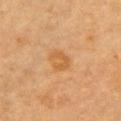Captured during whole-body skin photography for melanoma surveillance; the lesion was not biopsied. A roughly 15 mm field-of-view crop from a total-body skin photograph. The lesion is on the chest. This is a cross-polarized tile. About 2.5 mm across. The patient is a male aged approximately 85 or older.